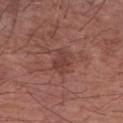Recorded during total-body skin imaging; not selected for excision or biopsy.
A male subject, aged 63–67.
A lesion tile, about 15 mm wide, cut from a 3D total-body photograph.
The lesion is on the arm.